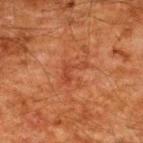No biopsy was performed on this lesion — it was imaged during a full skin examination and was not determined to be concerning. A 15 mm close-up tile from a total-body photography series done for melanoma screening. The total-body-photography lesion software estimated a lesion color around L≈35 a*≈26 b*≈30 in CIELAB, a lesion–skin lightness drop of about 6, and a lesion-to-skin contrast of about 5 (normalized; higher = more distinct). The software also gave border irregularity of about 8 on a 0–10 scale and a within-lesion color-variation index near 0/10. The subject is a male about 60 years old. Measured at roughly 3.5 mm in maximum diameter. The lesion is on the upper back. Imaged with cross-polarized lighting.